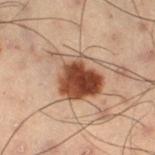biopsy_status: not biopsied; imaged during a skin examination
image:
  source: total-body photography crop
  field_of_view_mm: 15
patient:
  sex: male
  age_approx: 55
site: right thigh